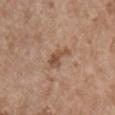Q: Is there a histopathology result?
A: imaged on a skin check; not biopsied
Q: Where on the body is the lesion?
A: the right upper arm
Q: What is the imaging modality?
A: total-body-photography crop, ~15 mm field of view
Q: Who is the patient?
A: female, in their mid- to late 60s
Q: Illumination type?
A: white-light
Q: How large is the lesion?
A: ~3.5 mm (longest diameter)